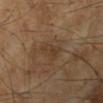Impression: Imaged during a routine full-body skin examination; the lesion was not biopsied and no histopathology is available. Image and clinical context: Longest diameter approximately 3.5 mm. A male subject aged approximately 65. A 15 mm crop from a total-body photograph taken for skin-cancer surveillance. On the left lower leg.